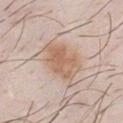Case summary:
* tile lighting: white-light illumination
* subject: male, about 30 years old
* acquisition: 15 mm crop, total-body photography
* location: the chest